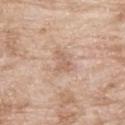No biopsy was performed on this lesion — it was imaged during a full skin examination and was not determined to be concerning. A male subject aged approximately 65. Longest diameter approximately 2.5 mm. Captured under white-light illumination. From the back. A close-up tile cropped from a whole-body skin photograph, about 15 mm across.